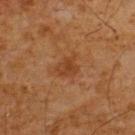* follow-up · no biopsy performed (imaged during a skin exam)
* imaging modality · total-body-photography crop, ~15 mm field of view
* site · the upper back
* patient · male, aged approximately 60